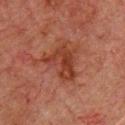No biopsy was performed on this lesion — it was imaged during a full skin examination and was not determined to be concerning. The lesion is on the chest. Cropped from a total-body skin-imaging series; the visible field is about 15 mm. The tile uses cross-polarized illumination. The patient is a male aged 58–62. An algorithmic analysis of the crop reported a lesion area of about 13 mm², an eccentricity of roughly 0.6, and a symmetry-axis asymmetry near 0.45. The software also gave a color-variation rating of about 4/10.